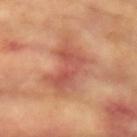No biopsy was performed on this lesion — it was imaged during a full skin examination and was not determined to be concerning.
A female subject, aged 73–77.
On the right upper arm.
This is a cross-polarized tile.
A region of skin cropped from a whole-body photographic capture, roughly 15 mm wide.
The lesion's longest dimension is about 8 mm.